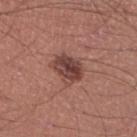workup — catalogued during a skin exam; not biopsied
tile lighting — white-light
automated metrics — an outline eccentricity of about 0.65 (0 = round, 1 = elongated) and a shape-asymmetry score of about 0.25 (0 = symmetric); an average lesion color of about L≈43 a*≈22 b*≈23 (CIELAB) and a lesion-to-skin contrast of about 9.5 (normalized; higher = more distinct); lesion-presence confidence of about 100/100
location — the leg
imaging modality — ~15 mm crop, total-body skin-cancer survey
lesion size — ≈3.5 mm
subject — male, aged approximately 45